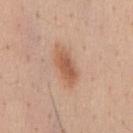Q: Was this lesion biopsied?
A: catalogued during a skin exam; not biopsied